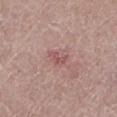– biopsy status · no biopsy performed (imaged during a skin exam)
– tile lighting · white-light illumination
– site · the leg
– acquisition · total-body-photography crop, ~15 mm field of view
– subject · male, in their 80s